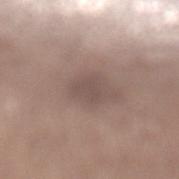This lesion was catalogued during total-body skin photography and was not selected for biopsy. A female patient, approximately 55 years of age. The lesion-visualizer software estimated a footprint of about 4 mm² and a shape-asymmetry score of about 0.45 (0 = symmetric). The analysis additionally found a nevus-likeness score of about 0/100 and lesion-presence confidence of about 95/100. On the left lower leg. A region of skin cropped from a whole-body photographic capture, roughly 15 mm wide. Captured under white-light illumination.